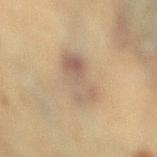Findings:
– workup — catalogued during a skin exam; not biopsied
– lighting — cross-polarized illumination
– body site — the right lower leg
– imaging modality — ~15 mm crop, total-body skin-cancer survey
– patient — female, aged 53 to 57
– size — ≈4.5 mm
– image-analysis metrics — a footprint of about 13 mm² and a symmetry-axis asymmetry near 0.25; a mean CIELAB color near L≈49 a*≈12 b*≈24 and roughly 8 lightness units darker than nearby skin; a border-irregularity rating of about 2.5/10 and a within-lesion color-variation index near 5.5/10; a classifier nevus-likeness of about 5/100 and a detector confidence of about 100 out of 100 that the crop contains a lesion